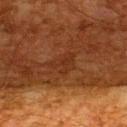Q: Was a biopsy performed?
A: total-body-photography surveillance lesion; no biopsy
Q: What is the imaging modality?
A: ~15 mm crop, total-body skin-cancer survey
Q: What is the anatomic site?
A: the upper back
Q: What are the patient's age and sex?
A: male, aged approximately 65